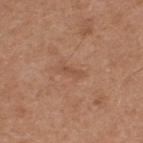Captured during whole-body skin photography for melanoma surveillance; the lesion was not biopsied.
Approximately 3 mm at its widest.
The lesion is located on the upper back.
A male subject roughly 65 years of age.
A 15 mm close-up tile from a total-body photography series done for melanoma screening.